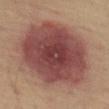Clinical summary:
Cropped from a total-body skin-imaging series; the visible field is about 15 mm. A male subject, in their mid-60s. Imaged with cross-polarized lighting. On the left thigh.